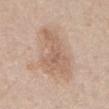Clinical impression:
Recorded during total-body skin imaging; not selected for excision or biopsy.